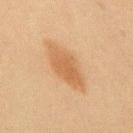  biopsy_status: not biopsied; imaged during a skin examination
  lighting: cross-polarized
  site: upper back
  patient:
    sex: male
    age_approx: 45
  image:
    source: total-body photography crop
    field_of_view_mm: 15